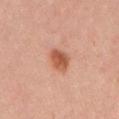biopsy_status: not biopsied; imaged during a skin examination
lighting: white-light
site: right upper arm
image:
  source: total-body photography crop
  field_of_view_mm: 15
patient:
  sex: female
  age_approx: 25
lesion_size:
  long_diameter_mm_approx: 3.0